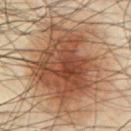- workup — catalogued during a skin exam; not biopsied
- lesion diameter — ~14.5 mm (longest diameter)
- location — the chest
- illumination — cross-polarized
- acquisition — total-body-photography crop, ~15 mm field of view
- subject — male, approximately 65 years of age
- image-analysis metrics — a footprint of about 95 mm²; a border-irregularity index near 6.5/10; a nevus-likeness score of about 95/100 and lesion-presence confidence of about 100/100